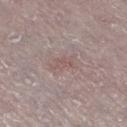Case summary:
– notes: no biopsy performed (imaged during a skin exam)
– image-analysis metrics: an area of roughly 3 mm², an outline eccentricity of about 0.85 (0 = round, 1 = elongated), and a symmetry-axis asymmetry near 0.45; an automated nevus-likeness rating near 0 out of 100 and lesion-presence confidence of about 80/100
– patient: female, roughly 65 years of age
– body site: the left lower leg
– size: about 2.5 mm
– image source: total-body-photography crop, ~15 mm field of view